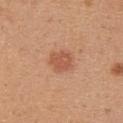Clinical impression:
The lesion was tiled from a total-body skin photograph and was not biopsied.
Acquisition and patient details:
From the upper back. The tile uses white-light illumination. A close-up tile cropped from a whole-body skin photograph, about 15 mm across. Automated image analysis of the tile measured a border-irregularity rating of about 2/10 and a color-variation rating of about 2/10. The analysis additionally found an automated nevus-likeness rating near 85 out of 100. A female patient roughly 25 years of age. Measured at roughly 3 mm in maximum diameter.